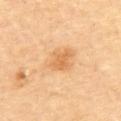site: the upper back; patient: male, approximately 85 years of age or older; lighting: cross-polarized illumination; lesion diameter: about 5 mm; image source: total-body-photography crop, ~15 mm field of view.